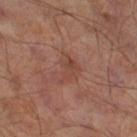Automated image analysis of the tile measured an average lesion color of about L≈42 a*≈21 b*≈26 (CIELAB) and about 6 CIELAB-L* units darker than the surrounding skin. Imaged with cross-polarized lighting. The lesion is located on the leg. A male patient, approximately 65 years of age. The lesion's longest dimension is about 3.5 mm. A region of skin cropped from a whole-body photographic capture, roughly 15 mm wide.